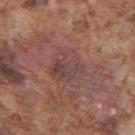follow-up: imaged on a skin check; not biopsied
site: the right upper arm
acquisition: ~15 mm crop, total-body skin-cancer survey
subject: male, roughly 75 years of age